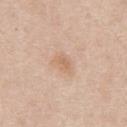Context:
An algorithmic analysis of the crop reported a mean CIELAB color near L≈65 a*≈19 b*≈32, a lesion–skin lightness drop of about 7, and a normalized border contrast of about 5.5. The software also gave a classifier nevus-likeness of about 10/100 and a detector confidence of about 100 out of 100 that the crop contains a lesion. Longest diameter approximately 2.5 mm. From the mid back. A lesion tile, about 15 mm wide, cut from a 3D total-body photograph. Captured under white-light illumination. The patient is a male approximately 60 years of age.